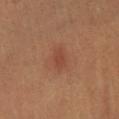Part of a total-body skin-imaging series; this lesion was reviewed on a skin check and was not flagged for biopsy. The lesion is on the left leg. The subject is a female in their mid- to late 40s. A region of skin cropped from a whole-body photographic capture, roughly 15 mm wide.